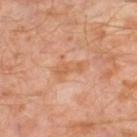Clinical impression:
Captured during whole-body skin photography for melanoma surveillance; the lesion was not biopsied.
Background:
A male patient, aged around 30. On the left thigh. A close-up tile cropped from a whole-body skin photograph, about 15 mm across. Automated tile analysis of the lesion measured a footprint of about 6.5 mm², a shape eccentricity near 0.85, and two-axis asymmetry of about 0.45. And it measured a mean CIELAB color near L≈59 a*≈23 b*≈36, roughly 6 lightness units darker than nearby skin, and a lesion-to-skin contrast of about 5.5 (normalized; higher = more distinct). It also reported border irregularity of about 6 on a 0–10 scale, internal color variation of about 2.5 on a 0–10 scale, and radial color variation of about 1. Measured at roughly 4 mm in maximum diameter. The tile uses cross-polarized illumination.